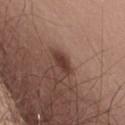notes: total-body-photography surveillance lesion; no biopsy
illumination: white-light
patient: male, in their mid- to late 50s
lesion diameter: about 3 mm
body site: the upper back
TBP lesion metrics: an area of roughly 3.5 mm² and a shape-asymmetry score of about 0.3 (0 = symmetric); a lesion–skin lightness drop of about 11 and a lesion-to-skin contrast of about 9.5 (normalized; higher = more distinct); internal color variation of about 1 on a 0–10 scale; a classifier nevus-likeness of about 90/100 and a lesion-detection confidence of about 100/100
acquisition: total-body-photography crop, ~15 mm field of view